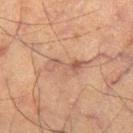patient=male, in their mid-60s
tile lighting=cross-polarized illumination
acquisition=~15 mm tile from a whole-body skin photo
body site=the left thigh
size=about 5 mm